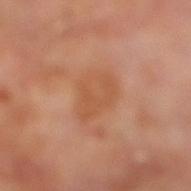notes: total-body-photography surveillance lesion; no biopsy | image source: total-body-photography crop, ~15 mm field of view | illumination: cross-polarized | subject: male, aged approximately 70 | diameter: ≈4.5 mm | location: the right lower leg | automated metrics: a lesion area of about 11 mm², an eccentricity of roughly 0.75, and a shape-asymmetry score of about 0.3 (0 = symmetric); a color-variation rating of about 2.5/10; a lesion-detection confidence of about 100/100.